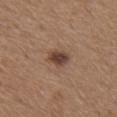notes = no biopsy performed (imaged during a skin exam)
patient = male, about 65 years old
location = the chest
image = ~15 mm tile from a whole-body skin photo